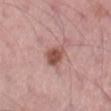Imaged with white-light lighting.
From the right thigh.
Approximately 3.5 mm at its widest.
Automated image analysis of the tile measured an average lesion color of about L≈52 a*≈23 b*≈25 (CIELAB), roughly 13 lightness units darker than nearby skin, and a lesion-to-skin contrast of about 9 (normalized; higher = more distinct). The analysis additionally found a border-irregularity index near 2.5/10, internal color variation of about 4 on a 0–10 scale, and peripheral color asymmetry of about 1.5.
A 15 mm close-up extracted from a 3D total-body photography capture.
A male subject aged around 55.